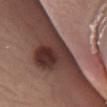Impression: Part of a total-body skin-imaging series; this lesion was reviewed on a skin check and was not flagged for biopsy. Background: A 15 mm close-up tile from a total-body photography series done for melanoma screening. The lesion is on the right lower leg. A male patient aged 33–37. Imaged with white-light lighting. The lesion's longest dimension is about 6.5 mm.